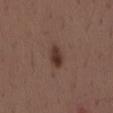This lesion was catalogued during total-body skin photography and was not selected for biopsy.
A male patient aged approximately 40.
From the lower back.
A 15 mm close-up tile from a total-body photography series done for melanoma screening.